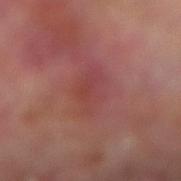{
  "biopsy_status": "not biopsied; imaged during a skin examination",
  "site": "right lower leg",
  "image": {
    "source": "total-body photography crop",
    "field_of_view_mm": 15
  },
  "automated_metrics": {
    "cielab_L": 42,
    "cielab_a": 27,
    "cielab_b": 23,
    "vs_skin_darker_L": 5.0,
    "vs_skin_contrast_norm": 4.5,
    "nevus_likeness_0_100": 0,
    "lesion_detection_confidence_0_100": 95
  },
  "lesion_size": {
    "long_diameter_mm_approx": 5.0
  },
  "patient": {
    "sex": "male",
    "age_approx": 70
  },
  "lighting": "cross-polarized"
}